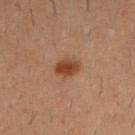The lesion was tiled from a total-body skin photograph and was not biopsied.
The lesion-visualizer software estimated a classifier nevus-likeness of about 100/100 and a lesion-detection confidence of about 100/100.
Captured under cross-polarized illumination.
Located on the left forearm.
The subject is a male aged approximately 30.
Approximately 3 mm at its widest.
A 15 mm crop from a total-body photograph taken for skin-cancer surveillance.